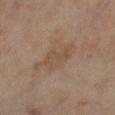biopsy_status: not biopsied; imaged during a skin examination
site: right lower leg
lighting: cross-polarized
image:
  source: total-body photography crop
  field_of_view_mm: 15
automated_metrics:
  area_mm2_approx: 8.0
  eccentricity: 0.9
  cielab_L: 42
  cielab_a: 13
  cielab_b: 25
  vs_skin_darker_L: 5.0
  vs_skin_contrast_norm: 5.0
  border_irregularity_0_10: 3.5
  color_variation_0_10: 1.5
  peripheral_color_asymmetry: 0.5
  nevus_likeness_0_100: 0
  lesion_detection_confidence_0_100: 100
lesion_size:
  long_diameter_mm_approx: 4.5
patient:
  sex: female
  age_approx: 55